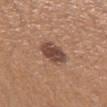Recorded during total-body skin imaging; not selected for excision or biopsy. From the arm. A 15 mm crop from a total-body photograph taken for skin-cancer surveillance. The patient is a female about 40 years old.